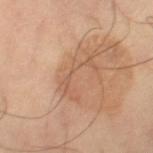Automated image analysis of the tile measured a footprint of about 6.5 mm² and two-axis asymmetry of about 0.8. And it measured border irregularity of about 10 on a 0–10 scale. About 5.5 mm across. A 15 mm close-up tile from a total-body photography series done for melanoma screening. The lesion is located on the right thigh. The patient is a male aged 58–62. This is a cross-polarized tile.